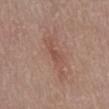notes: imaged on a skin check; not biopsied
subject: male, aged approximately 80
image: total-body-photography crop, ~15 mm field of view
body site: the abdomen